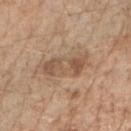biopsy status — total-body-photography surveillance lesion; no biopsy
body site — the right forearm
patient — male, approximately 70 years of age
lighting — white-light illumination
imaging modality — ~15 mm tile from a whole-body skin photo